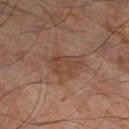This lesion was catalogued during total-body skin photography and was not selected for biopsy.
The tile uses cross-polarized illumination.
The patient is a male in their mid- to late 50s.
Longest diameter approximately 4.5 mm.
The lesion is located on the left lower leg.
Cropped from a whole-body photographic skin survey; the tile spans about 15 mm.